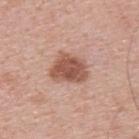follow-up — catalogued during a skin exam; not biopsied.